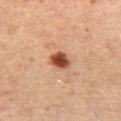Recorded during total-body skin imaging; not selected for excision or biopsy.
From the left thigh.
Imaged with cross-polarized lighting.
A female patient in their mid-40s.
Cropped from a total-body skin-imaging series; the visible field is about 15 mm.